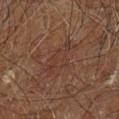Q: Was this lesion biopsied?
A: imaged on a skin check; not biopsied
Q: How large is the lesion?
A: ~5 mm (longest diameter)
Q: How was this image acquired?
A: total-body-photography crop, ~15 mm field of view
Q: Patient demographics?
A: male, aged 58 to 62
Q: What lighting was used for the tile?
A: cross-polarized
Q: What did automated image analysis measure?
A: a footprint of about 11 mm² and two-axis asymmetry of about 0.35; a mean CIELAB color near L≈35 a*≈18 b*≈24, a lesion–skin lightness drop of about 4, and a lesion-to-skin contrast of about 4.5 (normalized; higher = more distinct)
Q: What is the anatomic site?
A: the right leg